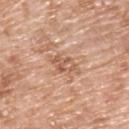Q: Was this lesion biopsied?
A: catalogued during a skin exam; not biopsied
Q: What kind of image is this?
A: ~15 mm crop, total-body skin-cancer survey
Q: Patient demographics?
A: male, in their mid- to late 70s
Q: What is the lesion's diameter?
A: about 3.5 mm
Q: What lighting was used for the tile?
A: white-light
Q: Where on the body is the lesion?
A: the upper back